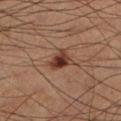Imaged during a routine full-body skin examination; the lesion was not biopsied and no histopathology is available. A male patient, aged 58 to 62. Cropped from a whole-body photographic skin survey; the tile spans about 15 mm. The lesion is on the leg.